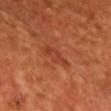Impression: This lesion was catalogued during total-body skin photography and was not selected for biopsy. Acquisition and patient details: The tile uses cross-polarized illumination. Longest diameter approximately 3.5 mm. From the chest. A close-up tile cropped from a whole-body skin photograph, about 15 mm across. Automated tile analysis of the lesion measured an area of roughly 4.5 mm² and two-axis asymmetry of about 0.3. A male patient aged approximately 50.